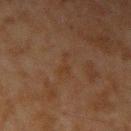Captured during whole-body skin photography for melanoma surveillance; the lesion was not biopsied. Approximately 3 mm at its widest. A 15 mm crop from a total-body photograph taken for skin-cancer surveillance. The lesion is located on the right upper arm. A male patient, approximately 45 years of age. Automated tile analysis of the lesion measured a lesion area of about 2.5 mm², an eccentricity of roughly 0.9, and two-axis asymmetry of about 0.6. The software also gave an automated nevus-likeness rating near 0 out of 100 and a lesion-detection confidence of about 100/100.